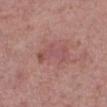Q: Was a biopsy performed?
A: imaged on a skin check; not biopsied
Q: Lesion size?
A: ≈4 mm
Q: How was the tile lit?
A: white-light
Q: What are the patient's age and sex?
A: female, aged 38 to 42
Q: What is the anatomic site?
A: the leg
Q: How was this image acquired?
A: ~15 mm crop, total-body skin-cancer survey